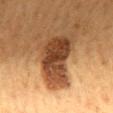{"biopsy_status": "not biopsied; imaged during a skin examination", "automated_metrics": {"eccentricity": 0.75, "shape_asymmetry": 0.3}, "lesion_size": {"long_diameter_mm_approx": 6.0}, "site": "mid back", "image": {"source": "total-body photography crop", "field_of_view_mm": 15}, "lighting": "cross-polarized", "patient": {"sex": "male", "age_approx": 60}}